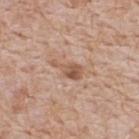notes: total-body-photography surveillance lesion; no biopsy
image-analysis metrics: a shape eccentricity near 0.9 and two-axis asymmetry of about 0.5; a nevus-likeness score of about 0/100
site: the upper back
lighting: white-light illumination
imaging modality: 15 mm crop, total-body photography
patient: male, aged 63–67
lesion size: ~4 mm (longest diameter)